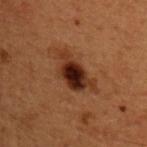The lesion was photographed on a routine skin check and not biopsied; there is no pathology result. A male patient, aged 48–52. On the upper back. Imaged with cross-polarized lighting. A lesion tile, about 15 mm wide, cut from a 3D total-body photograph. The lesion-visualizer software estimated an area of roughly 11 mm² and an outline eccentricity of about 0.85 (0 = round, 1 = elongated). The analysis additionally found a border-irregularity index near 4.5/10, a within-lesion color-variation index near 7.5/10, and a peripheral color-asymmetry measure near 1.5.